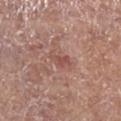Notes:
* biopsy status · imaged on a skin check; not biopsied
* subject · male, aged around 70
* site · the right lower leg
* image source · 15 mm crop, total-body photography